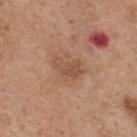follow-up: total-body-photography surveillance lesion; no biopsy
subject: male, about 65 years old
image source: 15 mm crop, total-body photography
size: about 3.5 mm
automated metrics: an outline eccentricity of about 0.8 (0 = round, 1 = elongated); a lesion–skin lightness drop of about 8 and a normalized lesion–skin contrast near 6; a classifier nevus-likeness of about 0/100 and lesion-presence confidence of about 100/100
body site: the upper back
illumination: white-light